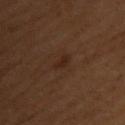The lesion was photographed on a routine skin check and not biopsied; there is no pathology result. Located on the chest. A female patient in their 50s. A 15 mm crop from a total-body photograph taken for skin-cancer surveillance.